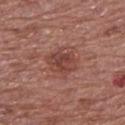Part of a total-body skin-imaging series; this lesion was reviewed on a skin check and was not flagged for biopsy. Automated tile analysis of the lesion measured a lesion area of about 8 mm², a shape eccentricity near 0.6, and two-axis asymmetry of about 0.15. It also reported a border-irregularity index near 2/10, a color-variation rating of about 4/10, and peripheral color asymmetry of about 1.5. This is a white-light tile. A 15 mm close-up tile from a total-body photography series done for melanoma screening. The lesion is on the upper back. Measured at roughly 4 mm in maximum diameter. A male patient, roughly 55 years of age.